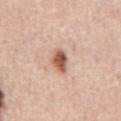Background: The lesion is located on the abdomen. A male patient, in their mid-60s. This is a white-light tile. Cropped from a whole-body photographic skin survey; the tile spans about 15 mm. The recorded lesion diameter is about 3 mm.